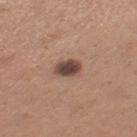workup: total-body-photography surveillance lesion; no biopsy | anatomic site: the leg | acquisition: ~15 mm tile from a whole-body skin photo | TBP lesion metrics: a lesion area of about 5.5 mm² and an eccentricity of roughly 0.65; a border-irregularity index near 1.5/10, a color-variation rating of about 4.5/10, and radial color variation of about 1; a nevus-likeness score of about 60/100 and a lesion-detection confidence of about 100/100 | subject: female, roughly 30 years of age | tile lighting: white-light illumination.